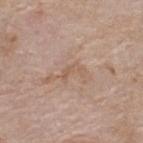{"biopsy_status": "not biopsied; imaged during a skin examination", "lesion_size": {"long_diameter_mm_approx": 2.5}, "site": "upper back", "lighting": "white-light", "patient": {"sex": "female", "age_approx": 75}, "image": {"source": "total-body photography crop", "field_of_view_mm": 15}}